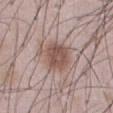subject: male, aged 48 to 52 | lighting: white-light | automated lesion analysis: a mean CIELAB color near L≈53 a*≈17 b*≈23 and a normalized lesion–skin contrast near 8; border irregularity of about 3 on a 0–10 scale, internal color variation of about 3.5 on a 0–10 scale, and radial color variation of about 1 | image source: 15 mm crop, total-body photography | site: the abdomen | lesion diameter: about 4.5 mm.